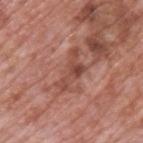<record>
<image>
  <source>total-body photography crop</source>
  <field_of_view_mm>15</field_of_view_mm>
</image>
<site>upper back</site>
<automated_metrics>
  <area_mm2_approx>8.5</area_mm2_approx>
  <eccentricity>0.9</eccentricity>
  <shape_asymmetry>0.45</shape_asymmetry>
  <cielab_L>48</cielab_L>
  <cielab_a>25</cielab_a>
  <cielab_b>27</cielab_b>
  <vs_skin_darker_L>9.0</vs_skin_darker_L>
  <border_irregularity_0_10>6.5</border_irregularity_0_10>
  <color_variation_0_10>4.5</color_variation_0_10>
  <peripheral_color_asymmetry>1.5</peripheral_color_asymmetry>
</automated_metrics>
<patient>
  <sex>male</sex>
  <age_approx>70</age_approx>
</patient>
<lighting>white-light</lighting>
<lesion_size>
  <long_diameter_mm_approx>5.5</long_diameter_mm_approx>
</lesion_size>
</record>